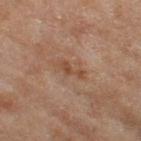Captured during whole-body skin photography for melanoma surveillance; the lesion was not biopsied.
A female patient, about 60 years old.
The lesion is located on the left thigh.
Longest diameter approximately 3.5 mm.
A 15 mm close-up tile from a total-body photography series done for melanoma screening.